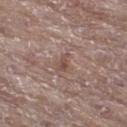No biopsy was performed on this lesion — it was imaged during a full skin examination and was not determined to be concerning. A region of skin cropped from a whole-body photographic capture, roughly 15 mm wide. On the left lower leg. The patient is a female approximately 85 years of age or older.